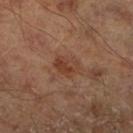Assessment: This lesion was catalogued during total-body skin photography and was not selected for biopsy. Image and clinical context: A male subject, roughly 65 years of age. Located on the right lower leg. Imaged with cross-polarized lighting. Cropped from a whole-body photographic skin survey; the tile spans about 15 mm.